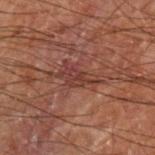Q: Was a biopsy performed?
A: no biopsy performed (imaged during a skin exam)
Q: Lesion location?
A: the left forearm
Q: How was this image acquired?
A: ~15 mm tile from a whole-body skin photo
Q: Who is the patient?
A: male, aged 68 to 72